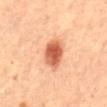Case summary:
• workup: no biopsy performed (imaged during a skin exam)
• TBP lesion metrics: a lesion area of about 9 mm², a shape eccentricity near 0.65, and a symmetry-axis asymmetry near 0.15; a mean CIELAB color near L≈54 a*≈27 b*≈34, about 15 CIELAB-L* units darker than the surrounding skin, and a normalized lesion–skin contrast near 10; a nevus-likeness score of about 100/100 and lesion-presence confidence of about 100/100
• tile lighting: cross-polarized
• site: the abdomen
• image: 15 mm crop, total-body photography
• size: about 4 mm
• patient: female, aged 58–62